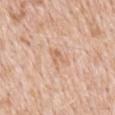workup=imaged on a skin check; not biopsied | automated lesion analysis=an average lesion color of about L≈66 a*≈20 b*≈33 (CIELAB) and about 7 CIELAB-L* units darker than the surrounding skin; a within-lesion color-variation index near 1/10 and radial color variation of about 0 | diameter=~3 mm (longest diameter) | body site=the back | image source=total-body-photography crop, ~15 mm field of view | illumination=white-light | patient=male, about 50 years old.